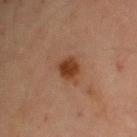<case>
<biopsy_status>not biopsied; imaged during a skin examination</biopsy_status>
<patient>
  <sex>female</sex>
  <age_approx>70</age_approx>
</patient>
<lesion_size>
  <long_diameter_mm_approx>3.0</long_diameter_mm_approx>
</lesion_size>
<image>
  <source>total-body photography crop</source>
  <field_of_view_mm>15</field_of_view_mm>
</image>
<automated_metrics>
  <eccentricity>0.65</eccentricity>
  <shape_asymmetry>0.25</shape_asymmetry>
  <cielab_L>34</cielab_L>
  <cielab_a>21</cielab_a>
  <cielab_b>29</cielab_b>
  <vs_skin_contrast_norm>10.0</vs_skin_contrast_norm>
  <color_variation_0_10>2.5</color_variation_0_10>
  <peripheral_color_asymmetry>1.0</peripheral_color_asymmetry>
</automated_metrics>
<site>right upper arm</site>
</case>